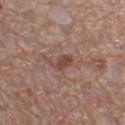Case summary:
• biopsy status — catalogued during a skin exam; not biopsied
• subject — male, roughly 70 years of age
• acquisition — total-body-photography crop, ~15 mm field of view
• site — the left thigh